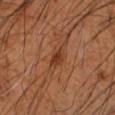| feature | finding |
|---|---|
| biopsy status | imaged on a skin check; not biopsied |
| patient | male, aged 58 to 62 |
| body site | the left forearm |
| acquisition | ~15 mm tile from a whole-body skin photo |